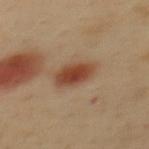| feature | finding |
|---|---|
| biopsy status | total-body-photography surveillance lesion; no biopsy |
| lighting | cross-polarized illumination |
| image | total-body-photography crop, ~15 mm field of view |
| subject | male, aged 38–42 |
| TBP lesion metrics | an average lesion color of about L≈45 a*≈22 b*≈31 (CIELAB) and about 12 CIELAB-L* units darker than the surrounding skin; internal color variation of about 4 on a 0–10 scale and radial color variation of about 1; a classifier nevus-likeness of about 100/100 |
| lesion size | about 4 mm |
| location | the upper back |